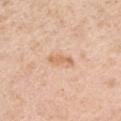Q: Is there a histopathology result?
A: catalogued during a skin exam; not biopsied
Q: How was the tile lit?
A: white-light illumination
Q: Lesion size?
A: ≈3.5 mm
Q: Where on the body is the lesion?
A: the right upper arm
Q: What are the patient's age and sex?
A: male, aged approximately 40
Q: How was this image acquired?
A: 15 mm crop, total-body photography